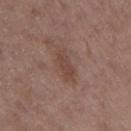{"biopsy_status": "not biopsied; imaged during a skin examination", "image": {"source": "total-body photography crop", "field_of_view_mm": 15}, "patient": {"sex": "male", "age_approx": 50}, "automated_metrics": {"area_mm2_approx": 5.5, "eccentricity": 0.85, "border_irregularity_0_10": 3.0, "peripheral_color_asymmetry": 0.5, "nevus_likeness_0_100": 5, "lesion_detection_confidence_0_100": 100}, "lesion_size": {"long_diameter_mm_approx": 3.5}, "lighting": "white-light", "site": "right upper arm"}